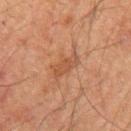Q: Was a biopsy performed?
A: no biopsy performed (imaged during a skin exam)
Q: Patient demographics?
A: male, aged around 65
Q: How was the tile lit?
A: cross-polarized
Q: How large is the lesion?
A: ~3.5 mm (longest diameter)
Q: How was this image acquired?
A: total-body-photography crop, ~15 mm field of view
Q: Where on the body is the lesion?
A: the left upper arm
Q: Automated lesion metrics?
A: a border-irregularity index near 3.5/10 and radial color variation of about 1; an automated nevus-likeness rating near 0 out of 100